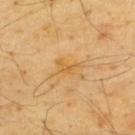Recorded during total-body skin imaging; not selected for excision or biopsy.
The lesion is on the upper back.
The patient is a male aged approximately 65.
The lesion-visualizer software estimated an area of roughly 4 mm², an outline eccentricity of about 0.8 (0 = round, 1 = elongated), and a symmetry-axis asymmetry near 0.35. The analysis additionally found a border-irregularity rating of about 4/10, a color-variation rating of about 2/10, and a peripheral color-asymmetry measure near 1.
Longest diameter approximately 3 mm.
This is a cross-polarized tile.
A roughly 15 mm field-of-view crop from a total-body skin photograph.